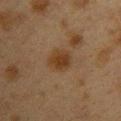Image and clinical context: A lesion tile, about 15 mm wide, cut from a 3D total-body photograph. The subject is a female in their 40s. Measured at roughly 3 mm in maximum diameter. Captured under cross-polarized illumination. The lesion is located on the upper back.